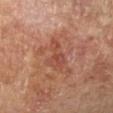| key | value |
|---|---|
| workup | imaged on a skin check; not biopsied |
| automated metrics | a footprint of about 7 mm², an outline eccentricity of about 0.9 (0 = round, 1 = elongated), and a shape-asymmetry score of about 0.45 (0 = symmetric); a lesion color around L≈47 a*≈25 b*≈30 in CIELAB and about 8 CIELAB-L* units darker than the surrounding skin; border irregularity of about 5.5 on a 0–10 scale, a color-variation rating of about 3/10, and peripheral color asymmetry of about 1 |
| tile lighting | cross-polarized |
| diameter | ~5 mm (longest diameter) |
| image source | 15 mm crop, total-body photography |
| subject | female, roughly 65 years of age |
| anatomic site | the arm |